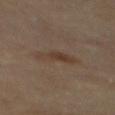Findings:
– follow-up · imaged on a skin check; not biopsied
– acquisition · ~15 mm crop, total-body skin-cancer survey
– diameter · ≈4 mm
– subject · male, aged 83 or over
– lighting · cross-polarized illumination
– site · the mid back